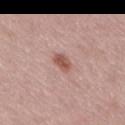<record>
  <biopsy_status>not biopsied; imaged during a skin examination</biopsy_status>
</record>